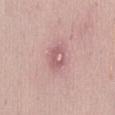workup: total-body-photography surveillance lesion; no biopsy
anatomic site: the abdomen
automated metrics: a lesion color around L≈59 a*≈24 b*≈19 in CIELAB, a lesion–skin lightness drop of about 9, and a normalized border contrast of about 6; peripheral color asymmetry of about 2.5; a classifier nevus-likeness of about 0/100 and lesion-presence confidence of about 95/100
subject: female, approximately 55 years of age
lighting: white-light illumination
lesion diameter: ~3 mm (longest diameter)
image source: 15 mm crop, total-body photography